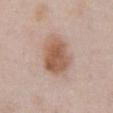The lesion was photographed on a routine skin check and not biopsied; there is no pathology result. Cropped from a whole-body photographic skin survey; the tile spans about 15 mm. The lesion is on the abdomen. The tile uses white-light illumination. A male patient, about 55 years old. An algorithmic analysis of the crop reported a mean CIELAB color near L≈57 a*≈19 b*≈29, about 12 CIELAB-L* units darker than the surrounding skin, and a normalized border contrast of about 8.5. It also reported border irregularity of about 1.5 on a 0–10 scale, a color-variation rating of about 4.5/10, and peripheral color asymmetry of about 1.5.